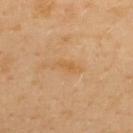Impression:
This lesion was catalogued during total-body skin photography and was not selected for biopsy.
Acquisition and patient details:
Automated tile analysis of the lesion measured a border-irregularity index near 2.5/10, a within-lesion color-variation index near 0.5/10, and a peripheral color-asymmetry measure near 0. Approximately 2.5 mm at its widest. The lesion is on the upper back. A 15 mm close-up extracted from a 3D total-body photography capture. A male subject, aged approximately 40.